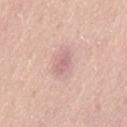Impression:
Captured during whole-body skin photography for melanoma surveillance; the lesion was not biopsied.
Acquisition and patient details:
The lesion is on the lower back. The patient is a male aged 48–52. Cropped from a whole-body photographic skin survey; the tile spans about 15 mm. Longest diameter approximately 3 mm. This is a white-light tile. The total-body-photography lesion software estimated a mean CIELAB color near L≈65 a*≈22 b*≈21 and roughly 9 lightness units darker than nearby skin. The software also gave border irregularity of about 2 on a 0–10 scale, internal color variation of about 1.5 on a 0–10 scale, and peripheral color asymmetry of about 0.5.